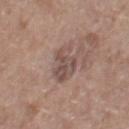Impression:
The lesion was photographed on a routine skin check and not biopsied; there is no pathology result.
Acquisition and patient details:
The lesion is on the right thigh. Cropped from a total-body skin-imaging series; the visible field is about 15 mm. The patient is a female aged 73 to 77. Approximately 4 mm at its widest. The tile uses white-light illumination.